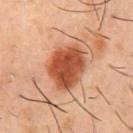Case summary:
- notes: catalogued during a skin exam; not biopsied
- location: the chest
- illumination: cross-polarized
- patient: male, about 55 years old
- image source: total-body-photography crop, ~15 mm field of view
- lesion size: about 5.5 mm